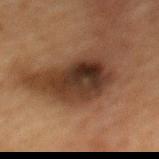| field | value |
|---|---|
| biopsy status | catalogued during a skin exam; not biopsied |
| lesion diameter | about 5.5 mm |
| imaging modality | total-body-photography crop, ~15 mm field of view |
| site | the mid back |
| patient | female, approximately 60 years of age |
| illumination | cross-polarized |